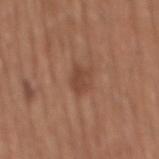biopsy status: imaged on a skin check; not biopsied
body site: the mid back
automated lesion analysis: a mean CIELAB color near L≈44 a*≈22 b*≈29, a lesion–skin lightness drop of about 8, and a lesion-to-skin contrast of about 6.5 (normalized; higher = more distinct); border irregularity of about 3 on a 0–10 scale, a color-variation rating of about 1.5/10, and peripheral color asymmetry of about 0.5; a nevus-likeness score of about 20/100 and lesion-presence confidence of about 100/100
tile lighting: white-light
image source: total-body-photography crop, ~15 mm field of view
patient: male, in their mid-60s